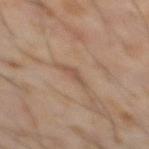Part of a total-body skin-imaging series; this lesion was reviewed on a skin check and was not flagged for biopsy.
This is a cross-polarized tile.
A 15 mm close-up tile from a total-body photography series done for melanoma screening.
The lesion is located on the abdomen.
The patient is a male aged approximately 60.